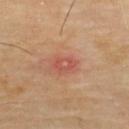anatomic site=the upper back | imaging modality=total-body-photography crop, ~15 mm field of view | patient=male, approximately 65 years of age | diameter=≈2.5 mm.